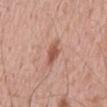Q: Was a biopsy performed?
A: catalogued during a skin exam; not biopsied
Q: Where on the body is the lesion?
A: the abdomen
Q: Lesion size?
A: ≈3 mm
Q: How was the tile lit?
A: white-light illumination
Q: What is the imaging modality?
A: ~15 mm crop, total-body skin-cancer survey
Q: Who is the patient?
A: male, aged approximately 60
Q: What did automated image analysis measure?
A: an automated nevus-likeness rating near 65 out of 100 and lesion-presence confidence of about 100/100